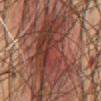biopsy_status: not biopsied; imaged during a skin examination
site: chest
lesion_size:
  long_diameter_mm_approx: 11.0
image:
  source: total-body photography crop
  field_of_view_mm: 15
patient:
  sex: male
  age_approx: 65
lighting: cross-polarized